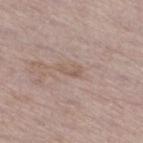Q: How was the tile lit?
A: white-light illumination
Q: What kind of image is this?
A: total-body-photography crop, ~15 mm field of view
Q: What are the patient's age and sex?
A: male, about 65 years old
Q: How large is the lesion?
A: ~2.5 mm (longest diameter)
Q: Lesion location?
A: the right thigh